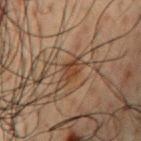workup: imaged on a skin check; not biopsied
acquisition: ~15 mm tile from a whole-body skin photo
site: the chest
TBP lesion metrics: an area of roughly 3.5 mm² and a shape-asymmetry score of about 0.25 (0 = symmetric); internal color variation of about 2 on a 0–10 scale and radial color variation of about 0.5
patient: male, aged 48–52
diameter: ~2.5 mm (longest diameter)